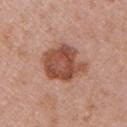Case summary:
– follow-up — catalogued during a skin exam; not biopsied